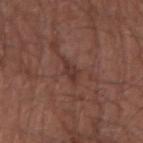The lesion was tiled from a total-body skin photograph and was not biopsied. From the right forearm. A male patient, aged 63–67. Longest diameter approximately 2.5 mm. A region of skin cropped from a whole-body photographic capture, roughly 15 mm wide. An algorithmic analysis of the crop reported a mean CIELAB color near L≈34 a*≈20 b*≈22 and a lesion–skin lightness drop of about 7. The analysis additionally found a border-irregularity index near 3.5/10 and a peripheral color-asymmetry measure near 0.5.